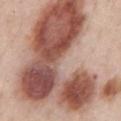<record>
<biopsy_status>not biopsied; imaged during a skin examination</biopsy_status>
<lighting>white-light</lighting>
<lesion_size>
  <long_diameter_mm_approx>15.0</long_diameter_mm_approx>
</lesion_size>
<patient>
  <sex>male</sex>
  <age_approx>50</age_approx>
</patient>
<site>front of the torso</site>
<image>
  <source>total-body photography crop</source>
  <field_of_view_mm>15</field_of_view_mm>
</image>
</record>